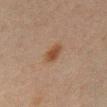Imaged during a routine full-body skin examination; the lesion was not biopsied and no histopathology is available.
Located on the back.
About 3 mm across.
A close-up tile cropped from a whole-body skin photograph, about 15 mm across.
The lesion-visualizer software estimated a border-irregularity rating of about 2/10, internal color variation of about 2.5 on a 0–10 scale, and radial color variation of about 0.5. And it measured a classifier nevus-likeness of about 95/100.
This is a cross-polarized tile.
The subject is a male about 70 years old.